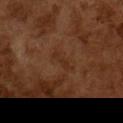Impression:
Recorded during total-body skin imaging; not selected for excision or biopsy.
Clinical summary:
The patient is a male aged 63 to 67. The lesion-visualizer software estimated a lesion area of about 2.5 mm² and an eccentricity of roughly 0.95. The analysis additionally found a classifier nevus-likeness of about 0/100 and a detector confidence of about 100 out of 100 that the crop contains a lesion. The recorded lesion diameter is about 2.5 mm. Imaged with cross-polarized lighting. Cropped from a total-body skin-imaging series; the visible field is about 15 mm.